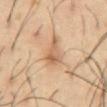This lesion was catalogued during total-body skin photography and was not selected for biopsy. A roughly 15 mm field-of-view crop from a total-body skin photograph. The lesion's longest dimension is about 4.5 mm. The lesion is located on the front of the torso. This is a cross-polarized tile. The patient is a male aged 53 to 57.